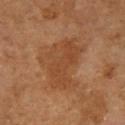This lesion was catalogued during total-body skin photography and was not selected for biopsy. From the right upper arm. A female patient aged 68–72. The lesion-visualizer software estimated a lesion area of about 23 mm², an outline eccentricity of about 0.7 (0 = round, 1 = elongated), and a shape-asymmetry score of about 0.45 (0 = symmetric). The analysis additionally found an average lesion color of about L≈43 a*≈21 b*≈34 (CIELAB) and roughly 7 lightness units darker than nearby skin. The analysis additionally found border irregularity of about 5 on a 0–10 scale and peripheral color asymmetry of about 1. It also reported an automated nevus-likeness rating near 10 out of 100 and lesion-presence confidence of about 100/100. The tile uses cross-polarized illumination. A 15 mm close-up extracted from a 3D total-body photography capture.